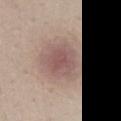<record>
  <biopsy_status>not biopsied; imaged during a skin examination</biopsy_status>
  <patient>
    <sex>female</sex>
    <age_approx>45</age_approx>
  </patient>
  <automated_metrics>
    <cielab_L>54</cielab_L>
    <cielab_a>18</cielab_a>
    <cielab_b>21</cielab_b>
    <vs_skin_darker_L>9.0</vs_skin_darker_L>
    <vs_skin_contrast_norm>6.5</vs_skin_contrast_norm>
  </automated_metrics>
  <site>lower back</site>
  <image>
    <source>total-body photography crop</source>
    <field_of_view_mm>15</field_of_view_mm>
  </image>
  <lesion_size>
    <long_diameter_mm_approx>4.5</long_diameter_mm_approx>
  </lesion_size>
</record>